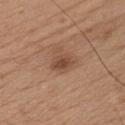Background:
The lesion's longest dimension is about 3 mm. This is a white-light tile. A male patient, aged 18–22. Cropped from a whole-body photographic skin survey; the tile spans about 15 mm. The lesion is located on the chest.